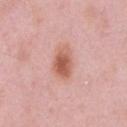| feature | finding |
|---|---|
| follow-up | imaged on a skin check; not biopsied |
| tile lighting | white-light |
| site | the chest |
| size | ≈4 mm |
| image | total-body-photography crop, ~15 mm field of view |
| subject | male, in their mid- to late 50s |
| automated lesion analysis | a footprint of about 8.5 mm² and a symmetry-axis asymmetry near 0.2; a lesion color around L≈59 a*≈25 b*≈29 in CIELAB, roughly 12 lightness units darker than nearby skin, and a normalized lesion–skin contrast near 8.5; a border-irregularity index near 2/10, a color-variation rating of about 5.5/10, and radial color variation of about 1.5; a nevus-likeness score of about 95/100 and lesion-presence confidence of about 100/100 |